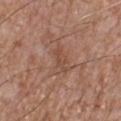Case summary:
– notes · total-body-photography surveillance lesion; no biopsy
– image · 15 mm crop, total-body photography
– tile lighting · white-light
– patient · male, aged around 75
– lesion size · ~3 mm (longest diameter)
– location · the right lower leg